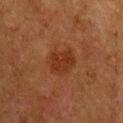notes: imaged on a skin check; not biopsied
subject: female, about 50 years old
body site: the chest
imaging modality: 15 mm crop, total-body photography